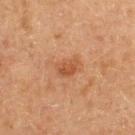Assessment:
Recorded during total-body skin imaging; not selected for excision or biopsy.
Clinical summary:
A close-up tile cropped from a whole-body skin photograph, about 15 mm across. The tile uses cross-polarized illumination. A male subject, aged around 60. The total-body-photography lesion software estimated about 8 CIELAB-L* units darker than the surrounding skin and a normalized lesion–skin contrast near 6.5. The software also gave a border-irregularity index near 3/10, a color-variation rating of about 2.5/10, and a peripheral color-asymmetry measure near 1. And it measured a classifier nevus-likeness of about 25/100 and a detector confidence of about 100 out of 100 that the crop contains a lesion. Measured at roughly 3 mm in maximum diameter.